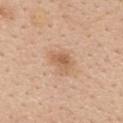No biopsy was performed on this lesion — it was imaged during a full skin examination and was not determined to be concerning. A female subject about 45 years old. Longest diameter approximately 3 mm. Automated image analysis of the tile measured a lesion area of about 5.5 mm² and a shape-asymmetry score of about 0.2 (0 = symmetric). The analysis additionally found a lesion color around L≈60 a*≈20 b*≈35 in CIELAB, roughly 9 lightness units darker than nearby skin, and a normalized lesion–skin contrast near 6.5. And it measured a border-irregularity rating of about 2/10 and internal color variation of about 3 on a 0–10 scale. And it measured an automated nevus-likeness rating near 45 out of 100 and a lesion-detection confidence of about 100/100. From the upper back. Cropped from a total-body skin-imaging series; the visible field is about 15 mm.